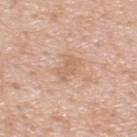site: the upper back; acquisition: total-body-photography crop, ~15 mm field of view; diameter: ≈3 mm; tile lighting: white-light illumination; patient: male, aged approximately 50.